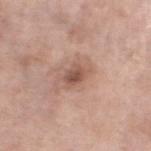Q: Was this lesion biopsied?
A: total-body-photography surveillance lesion; no biopsy
Q: What is the imaging modality?
A: total-body-photography crop, ~15 mm field of view
Q: Lesion location?
A: the left lower leg
Q: What are the patient's age and sex?
A: female, roughly 55 years of age